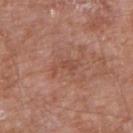Findings:
• follow-up: total-body-photography surveillance lesion; no biopsy
• imaging modality: total-body-photography crop, ~15 mm field of view
• location: the right upper arm
• subject: male, in their 80s